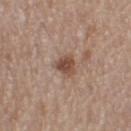{
  "biopsy_status": "not biopsied; imaged during a skin examination",
  "image": {
    "source": "total-body photography crop",
    "field_of_view_mm": 15
  },
  "lighting": "white-light",
  "patient": {
    "sex": "female",
    "age_approx": 40
  },
  "automated_metrics": {
    "area_mm2_approx": 5.0,
    "eccentricity": 0.55,
    "shape_asymmetry": 0.35,
    "border_irregularity_0_10": 3.0,
    "color_variation_0_10": 3.5,
    "peripheral_color_asymmetry": 1.0
  },
  "site": "left thigh",
  "lesion_size": {
    "long_diameter_mm_approx": 3.0
  }
}